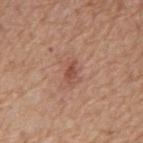Captured during whole-body skin photography for melanoma surveillance; the lesion was not biopsied.
The lesion is located on the mid back.
The patient is a male about 65 years old.
The tile uses white-light illumination.
The lesion's longest dimension is about 2.5 mm.
A roughly 15 mm field-of-view crop from a total-body skin photograph.